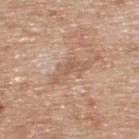| key | value |
|---|---|
| location | the upper back |
| lighting | white-light illumination |
| imaging modality | ~15 mm tile from a whole-body skin photo |
| image-analysis metrics | internal color variation of about 1.5 on a 0–10 scale and a peripheral color-asymmetry measure near 0.5; a classifier nevus-likeness of about 0/100 and lesion-presence confidence of about 95/100 |
| subject | male, approximately 60 years of age |